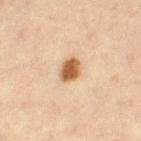notes = imaged on a skin check; not biopsied
location = the leg
imaging modality = 15 mm crop, total-body photography
subject = female, in their mid- to late 40s
TBP lesion metrics = a classifier nevus-likeness of about 100/100 and a detector confidence of about 100 out of 100 that the crop contains a lesion
tile lighting = cross-polarized